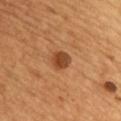Recorded during total-body skin imaging; not selected for excision or biopsy. The lesion is located on the chest. The lesion-visualizer software estimated a lesion area of about 5 mm² and a symmetry-axis asymmetry near 0.2. The analysis additionally found an average lesion color of about L≈45 a*≈25 b*≈37 (CIELAB), a lesion–skin lightness drop of about 12, and a normalized lesion–skin contrast near 9. The analysis additionally found border irregularity of about 1.5 on a 0–10 scale, a color-variation rating of about 4.5/10, and peripheral color asymmetry of about 1.5. A close-up tile cropped from a whole-body skin photograph, about 15 mm across. The recorded lesion diameter is about 2.5 mm. A male patient in their mid-40s.